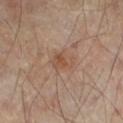The lesion was tiled from a total-body skin photograph and was not biopsied. Located on the left lower leg. The subject is a male approximately 65 years of age. This image is a 15 mm lesion crop taken from a total-body photograph. This is a cross-polarized tile. About 3 mm across.